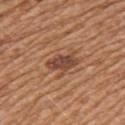tile lighting: white-light illumination
image-analysis metrics: an area of roughly 7 mm² and an outline eccentricity of about 0.85 (0 = round, 1 = elongated); a color-variation rating of about 3.5/10 and peripheral color asymmetry of about 1; an automated nevus-likeness rating near 45 out of 100 and lesion-presence confidence of about 100/100
location: the left upper arm
lesion size: ~4 mm (longest diameter)
patient: male, approximately 65 years of age
acquisition: total-body-photography crop, ~15 mm field of view